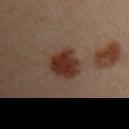Captured during whole-body skin photography for melanoma surveillance; the lesion was not biopsied. The subject is a female aged approximately 30. On the left arm. About 4 mm across. An algorithmic analysis of the crop reported a footprint of about 9 mm², an outline eccentricity of about 0.6 (0 = round, 1 = elongated), and two-axis asymmetry of about 0.2. And it measured a lesion color around L≈24 a*≈17 b*≈21 in CIELAB, about 10 CIELAB-L* units darker than the surrounding skin, and a normalized lesion–skin contrast near 11.5. The analysis additionally found a nevus-likeness score of about 95/100 and a lesion-detection confidence of about 100/100. A region of skin cropped from a whole-body photographic capture, roughly 15 mm wide.